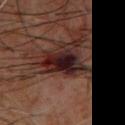Imaged during a routine full-body skin examination; the lesion was not biopsied and no histopathology is available. The subject is a male about 60 years old. Cropped from a total-body skin-imaging series; the visible field is about 15 mm. On the upper back.